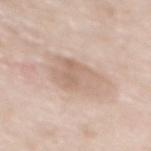Q: Is there a histopathology result?
A: no biopsy performed (imaged during a skin exam)
Q: Where on the body is the lesion?
A: the mid back
Q: Patient demographics?
A: female, aged around 75
Q: What did automated image analysis measure?
A: an area of roughly 15 mm², an outline eccentricity of about 0.8 (0 = round, 1 = elongated), and a shape-asymmetry score of about 0.25 (0 = symmetric); about 8 CIELAB-L* units darker than the surrounding skin and a normalized lesion–skin contrast near 5.5; a within-lesion color-variation index near 4/10 and radial color variation of about 1.5; a nevus-likeness score of about 0/100 and a detector confidence of about 95 out of 100 that the crop contains a lesion
Q: How was the tile lit?
A: white-light illumination
Q: What kind of image is this?
A: total-body-photography crop, ~15 mm field of view
Q: Lesion size?
A: about 6 mm